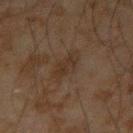The lesion was photographed on a routine skin check and not biopsied; there is no pathology result. This image is a 15 mm lesion crop taken from a total-body photograph. Captured under cross-polarized illumination. Automated image analysis of the tile measured an average lesion color of about L≈26 a*≈11 b*≈21 (CIELAB), a lesion–skin lightness drop of about 4, and a lesion-to-skin contrast of about 5.5 (normalized; higher = more distinct). The software also gave a border-irregularity index near 3.5/10, a within-lesion color-variation index near 2.5/10, and peripheral color asymmetry of about 1. The analysis additionally found an automated nevus-likeness rating near 0 out of 100 and lesion-presence confidence of about 100/100. The subject is a male aged 43 to 47. The recorded lesion diameter is about 4 mm. Located on the left forearm.